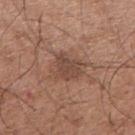Case summary:
– patient — male, in their 50s
– image source — 15 mm crop, total-body photography
– illumination — white-light
– location — the right upper arm
– image-analysis metrics — an outline eccentricity of about 0.65 (0 = round, 1 = elongated) and two-axis asymmetry of about 0.3; a border-irregularity rating of about 3/10, a within-lesion color-variation index near 3/10, and a peripheral color-asymmetry measure near 1